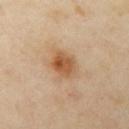Notes:
– workup · catalogued during a skin exam; not biopsied
– location · the arm
– imaging modality · ~15 mm crop, total-body skin-cancer survey
– patient · female, aged 38–42